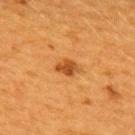subject: female, aged 38–42
illumination: cross-polarized
body site: the back
automated metrics: an area of roughly 4 mm², an outline eccentricity of about 0.7 (0 = round, 1 = elongated), and two-axis asymmetry of about 0.25; a mean CIELAB color near L≈41 a*≈24 b*≈40, about 10 CIELAB-L* units darker than the surrounding skin, and a normalized lesion–skin contrast near 8.5; a classifier nevus-likeness of about 90/100 and lesion-presence confidence of about 100/100
image: total-body-photography crop, ~15 mm field of view
lesion diameter: about 2.5 mm